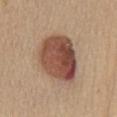workup: total-body-photography surveillance lesion; no biopsy | imaging modality: ~15 mm tile from a whole-body skin photo | automated lesion analysis: roughly 16 lightness units darker than nearby skin and a normalized lesion–skin contrast near 11; a border-irregularity rating of about 1.5/10 and peripheral color asymmetry of about 2.5; a classifier nevus-likeness of about 100/100 and lesion-presence confidence of about 100/100 | patient: female, roughly 60 years of age | site: the chest | tile lighting: white-light.